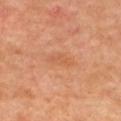No biopsy was performed on this lesion — it was imaged during a full skin examination and was not determined to be concerning.
Imaged with cross-polarized lighting.
Located on the upper back.
Cropped from a total-body skin-imaging series; the visible field is about 15 mm.
A male patient aged 58–62.
Approximately 3.5 mm at its widest.